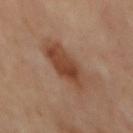Q: Was a biopsy performed?
A: imaged on a skin check; not biopsied
Q: What is the imaging modality?
A: ~15 mm tile from a whole-body skin photo
Q: How large is the lesion?
A: ~6.5 mm (longest diameter)
Q: What lighting was used for the tile?
A: cross-polarized illumination
Q: What is the anatomic site?
A: the mid back